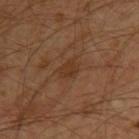biopsy_status: not biopsied; imaged during a skin examination
image:
  source: total-body photography crop
  field_of_view_mm: 15
patient:
  sex: male
  age_approx: 30
automated_metrics:
  eccentricity: 0.6
  shape_asymmetry: 0.25
  nevus_likeness_0_100: 0
  lesion_detection_confidence_0_100: 100
lesion_size:
  long_diameter_mm_approx: 2.5
site: left upper arm